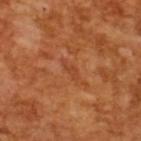The recorded lesion diameter is about 2.5 mm. A male patient in their mid-60s. Captured under cross-polarized illumination. A 15 mm close-up tile from a total-body photography series done for melanoma screening.